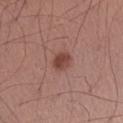biopsy status: total-body-photography surveillance lesion; no biopsy
diameter: ≈2.5 mm
location: the left forearm
image source: ~15 mm crop, total-body skin-cancer survey
patient: male, aged 33–37
illumination: white-light
automated lesion analysis: an eccentricity of roughly 0.6 and a shape-asymmetry score of about 0.25 (0 = symmetric); a lesion–skin lightness drop of about 11 and a normalized lesion–skin contrast near 8.5